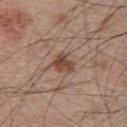biopsy status — catalogued during a skin exam; not biopsied | image source — ~15 mm crop, total-body skin-cancer survey | subject — male, roughly 65 years of age | location — the upper back.